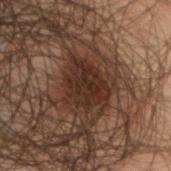No biopsy was performed on this lesion — it was imaged during a full skin examination and was not determined to be concerning. The patient is a female aged 18–22. Automated tile analysis of the lesion measured a footprint of about 14 mm², a shape eccentricity near 0.5, and a symmetry-axis asymmetry near 0.3. And it measured a border-irregularity index near 3/10, a color-variation rating of about 3.5/10, and a peripheral color-asymmetry measure near 1.5. Imaged with cross-polarized lighting. A 15 mm crop from a total-body photograph taken for skin-cancer surveillance. Approximately 5 mm at its widest. From the head or neck.